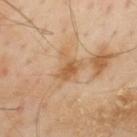Part of a total-body skin-imaging series; this lesion was reviewed on a skin check and was not flagged for biopsy.
This image is a 15 mm lesion crop taken from a total-body photograph.
Measured at roughly 3 mm in maximum diameter.
This is a cross-polarized tile.
A male subject aged 53–57.
Located on the back.